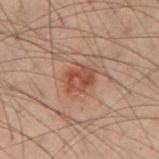Case summary:
• automated metrics · an area of roughly 7 mm² and an outline eccentricity of about 0.65 (0 = round, 1 = elongated); roughly 8 lightness units darker than nearby skin and a normalized border contrast of about 7.5; a within-lesion color-variation index near 4.5/10 and peripheral color asymmetry of about 1.5; a classifier nevus-likeness of about 25/100 and a detector confidence of about 100 out of 100 that the crop contains a lesion
• lesion diameter · ~3.5 mm (longest diameter)
• image · 15 mm crop, total-body photography
• subject · male, aged 38 to 42
• anatomic site · the right forearm
• tile lighting · cross-polarized